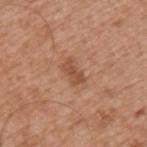Assessment:
The lesion was tiled from a total-body skin photograph and was not biopsied.
Background:
The recorded lesion diameter is about 3 mm. A lesion tile, about 15 mm wide, cut from a 3D total-body photograph. The subject is a male aged 53–57. Automated tile analysis of the lesion measured an area of roughly 4 mm², an outline eccentricity of about 0.85 (0 = round, 1 = elongated), and two-axis asymmetry of about 0.25. It also reported an automated nevus-likeness rating near 20 out of 100. Imaged with white-light lighting. The lesion is on the arm.